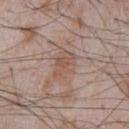| field | value |
|---|---|
| biopsy status | no biopsy performed (imaged during a skin exam) |
| location | the chest |
| lesion diameter | ≈3.5 mm |
| imaging modality | ~15 mm crop, total-body skin-cancer survey |
| lighting | white-light illumination |
| automated lesion analysis | a footprint of about 7.5 mm² and an eccentricity of roughly 0.75; a normalized lesion–skin contrast near 5.5; a border-irregularity index near 2/10, internal color variation of about 3 on a 0–10 scale, and a peripheral color-asymmetry measure near 1; an automated nevus-likeness rating near 0 out of 100 and a detector confidence of about 100 out of 100 that the crop contains a lesion |
| patient | male, aged 63–67 |